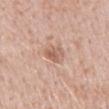Impression:
Part of a total-body skin-imaging series; this lesion was reviewed on a skin check and was not flagged for biopsy.
Context:
Longest diameter approximately 2.5 mm. The lesion is located on the mid back. This is a white-light tile. A roughly 15 mm field-of-view crop from a total-body skin photograph. The total-body-photography lesion software estimated a lesion color around L≈60 a*≈21 b*≈28 in CIELAB and a lesion-to-skin contrast of about 6.5 (normalized; higher = more distinct). And it measured an automated nevus-likeness rating near 10 out of 100. A male subject, aged 48–52.